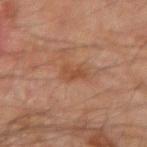Context: About 2.5 mm across. A male subject, about 45 years old. This is a cross-polarized tile. A lesion tile, about 15 mm wide, cut from a 3D total-body photograph. From the left forearm.